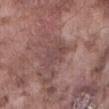Notes:
* workup · catalogued during a skin exam; not biopsied
* site · the front of the torso
* automated metrics · roughly 7 lightness units darker than nearby skin and a lesion-to-skin contrast of about 5.5 (normalized; higher = more distinct); a border-irregularity rating of about 4/10, a color-variation rating of about 3.5/10, and a peripheral color-asymmetry measure near 1
* size · about 4 mm
* acquisition · ~15 mm crop, total-body skin-cancer survey
* patient · male, aged 73–77
* tile lighting · white-light